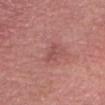Q: Was a biopsy performed?
A: catalogued during a skin exam; not biopsied
Q: Illumination type?
A: white-light
Q: What kind of image is this?
A: 15 mm crop, total-body photography
Q: Patient demographics?
A: male, aged 58 to 62
Q: Lesion size?
A: ~2.5 mm (longest diameter)
Q: What is the anatomic site?
A: the head or neck
Q: What did automated image analysis measure?
A: an average lesion color of about L≈50 a*≈26 b*≈24 (CIELAB) and a lesion-to-skin contrast of about 5 (normalized; higher = more distinct); border irregularity of about 5 on a 0–10 scale, a color-variation rating of about 0/10, and peripheral color asymmetry of about 0; lesion-presence confidence of about 100/100